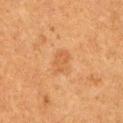Imaged during a routine full-body skin examination; the lesion was not biopsied and no histopathology is available. The recorded lesion diameter is about 3 mm. The lesion is on the left upper arm. A lesion tile, about 15 mm wide, cut from a 3D total-body photograph. A male patient, about 50 years old. Automated image analysis of the tile measured a shape eccentricity near 0.8. And it measured a classifier nevus-likeness of about 0/100.